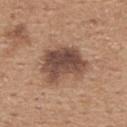Clinical impression:
This lesion was catalogued during total-body skin photography and was not selected for biopsy.
Image and clinical context:
A region of skin cropped from a whole-body photographic capture, roughly 15 mm wide. The subject is a male aged approximately 65. The tile uses white-light illumination. On the upper back. The lesion's longest dimension is about 6 mm.